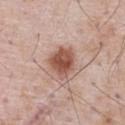follow-up: total-body-photography surveillance lesion; no biopsy | image: 15 mm crop, total-body photography | patient: male, in their 70s | image-analysis metrics: a footprint of about 10 mm² and a shape eccentricity near 0.55; a lesion color around L≈53 a*≈22 b*≈27 in CIELAB and a normalized lesion–skin contrast near 10; internal color variation of about 4 on a 0–10 scale and a peripheral color-asymmetry measure near 1 | size: ~3.5 mm (longest diameter) | location: the front of the torso.